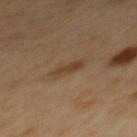workup: catalogued during a skin exam; not biopsied | size: ≈3.5 mm | body site: the back | image: 15 mm crop, total-body photography | tile lighting: cross-polarized illumination | TBP lesion metrics: a lesion area of about 5 mm², an outline eccentricity of about 0.9 (0 = round, 1 = elongated), and a symmetry-axis asymmetry near 0.25; a mean CIELAB color near L≈41 a*≈16 b*≈31, about 7 CIELAB-L* units darker than the surrounding skin, and a normalized lesion–skin contrast near 6.5; a border-irregularity rating of about 3/10, a within-lesion color-variation index near 2/10, and peripheral color asymmetry of about 0.5; lesion-presence confidence of about 100/100 | subject: male, aged approximately 50.